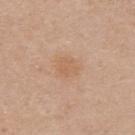workup: no biopsy performed (imaged during a skin exam) | patient: female, approximately 40 years of age | image: ~15 mm crop, total-body skin-cancer survey | site: the upper back | tile lighting: white-light illumination | lesion size: about 3 mm.